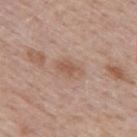notes = no biopsy performed (imaged during a skin exam) | site = the mid back | patient = male, roughly 40 years of age | acquisition = total-body-photography crop, ~15 mm field of view.